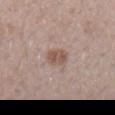No biopsy was performed on this lesion — it was imaged during a full skin examination and was not determined to be concerning.